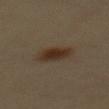Notes:
– notes — total-body-photography surveillance lesion; no biopsy
– anatomic site — the mid back
– lesion diameter — ≈4.5 mm
– acquisition — ~15 mm tile from a whole-body skin photo
– patient — male, approximately 40 years of age
– tile lighting — cross-polarized illumination